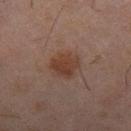Assessment:
No biopsy was performed on this lesion — it was imaged during a full skin examination and was not determined to be concerning.
Context:
Located on the right thigh. A lesion tile, about 15 mm wide, cut from a 3D total-body photograph. A male subject, aged 43–47. Measured at roughly 4 mm in maximum diameter.